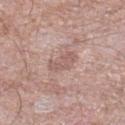The lesion was tiled from a total-body skin photograph and was not biopsied.
A roughly 15 mm field-of-view crop from a total-body skin photograph.
The lesion's longest dimension is about 3.5 mm.
Imaged with white-light lighting.
Automated image analysis of the tile measured a mean CIELAB color near L≈57 a*≈19 b*≈23, roughly 8 lightness units darker than nearby skin, and a normalized border contrast of about 5.5. The software also gave a border-irregularity index near 5/10 and a within-lesion color-variation index near 1.5/10.
A male patient aged around 60.
The lesion is located on the right lower leg.